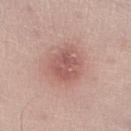Recorded during total-body skin imaging; not selected for excision or biopsy. A lesion tile, about 15 mm wide, cut from a 3D total-body photograph. The lesion's longest dimension is about 4.5 mm. A male subject aged 58 to 62. Captured under white-light illumination. On the right lower leg.